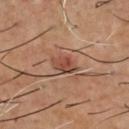biopsy status: total-body-photography surveillance lesion; no biopsy
patient: male, aged around 55
automated metrics: an eccentricity of roughly 0.75 and a shape-asymmetry score of about 0.25 (0 = symmetric); a mean CIELAB color near L≈45 a*≈21 b*≈28, a lesion–skin lightness drop of about 9, and a normalized lesion–skin contrast near 7.5
illumination: cross-polarized
body site: the front of the torso
acquisition: 15 mm crop, total-body photography